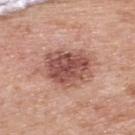The lesion's longest dimension is about 5.5 mm.
Captured under white-light illumination.
Cropped from a total-body skin-imaging series; the visible field is about 15 mm.
From the upper back.
A male patient aged approximately 70.